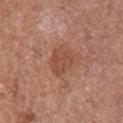Case summary:
– notes: no biopsy performed (imaged during a skin exam)
– tile lighting: white-light illumination
– patient: female, aged approximately 55
– lesion diameter: ~3.5 mm (longest diameter)
– image source: 15 mm crop, total-body photography
– location: the arm